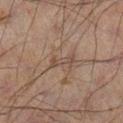Clinical impression:
The lesion was tiled from a total-body skin photograph and was not biopsied.
Background:
The recorded lesion diameter is about 3 mm. A male patient aged around 60. The total-body-photography lesion software estimated an area of roughly 2.5 mm², an outline eccentricity of about 0.95 (0 = round, 1 = elongated), and two-axis asymmetry of about 0.35. It also reported a lesion color around L≈34 a*≈13 b*≈20 in CIELAB, roughly 6 lightness units darker than nearby skin, and a normalized lesion–skin contrast near 5.5. Captured under cross-polarized illumination. A region of skin cropped from a whole-body photographic capture, roughly 15 mm wide. The lesion is on the left leg.